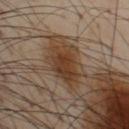{"site": "front of the torso", "lesion_size": {"long_diameter_mm_approx": 4.5}, "image": {"source": "total-body photography crop", "field_of_view_mm": 15}, "automated_metrics": {"area_mm2_approx": 11.0, "eccentricity": 0.7, "shape_asymmetry": 0.25, "lesion_detection_confidence_0_100": 100}, "patient": {"sex": "male", "age_approx": 55}, "lighting": "cross-polarized"}